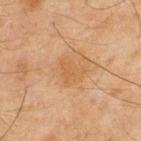A 15 mm crop from a total-body photograph taken for skin-cancer surveillance.
The total-body-photography lesion software estimated an average lesion color of about L≈48 a*≈18 b*≈34 (CIELAB) and roughly 5 lightness units darker than nearby skin. The software also gave a classifier nevus-likeness of about 0/100.
Captured under cross-polarized illumination.
The lesion is located on the back.
A male subject aged approximately 45.
Longest diameter approximately 3 mm.